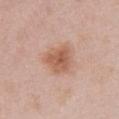{
  "image": {
    "source": "total-body photography crop",
    "field_of_view_mm": 15
  },
  "patient": {
    "sex": "female",
    "age_approx": 50
  },
  "site": "front of the torso",
  "lighting": "white-light",
  "lesion_size": {
    "long_diameter_mm_approx": 4.0
  },
  "automated_metrics": {
    "cielab_L": 59,
    "cielab_a": 21,
    "cielab_b": 31,
    "vs_skin_darker_L": 10.0,
    "vs_skin_contrast_norm": 7.5,
    "border_irregularity_0_10": 2.5,
    "nevus_likeness_0_100": 65,
    "lesion_detection_confidence_0_100": 100
  }
}